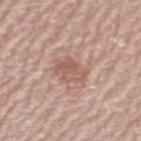This lesion was catalogued during total-body skin photography and was not selected for biopsy. Measured at roughly 3.5 mm in maximum diameter. The lesion is on the left upper arm. The patient is a female in their mid- to late 60s. A roughly 15 mm field-of-view crop from a total-body skin photograph.